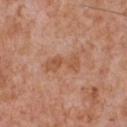{"biopsy_status": "not biopsied; imaged during a skin examination", "site": "chest", "patient": {"sex": "male", "age_approx": 65}, "image": {"source": "total-body photography crop", "field_of_view_mm": 15}, "lighting": "white-light"}